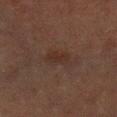Part of a total-body skin-imaging series; this lesion was reviewed on a skin check and was not flagged for biopsy.